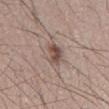Findings:
* tile lighting · white-light illumination
* location · the mid back
* diameter · ~4 mm (longest diameter)
* patient · male, approximately 45 years of age
* image-analysis metrics · border irregularity of about 3 on a 0–10 scale, internal color variation of about 4.5 on a 0–10 scale, and peripheral color asymmetry of about 1.5
* imaging modality · ~15 mm tile from a whole-body skin photo